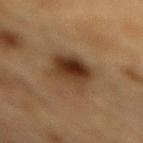Impression:
Imaged during a routine full-body skin examination; the lesion was not biopsied and no histopathology is available.
Context:
The recorded lesion diameter is about 4.5 mm. A region of skin cropped from a whole-body photographic capture, roughly 15 mm wide. From the mid back. The subject is a male aged approximately 85 or older. Captured under cross-polarized illumination. The total-body-photography lesion software estimated a lesion area of about 14 mm², a shape eccentricity near 0.45, and a shape-asymmetry score of about 0.25 (0 = symmetric). And it measured a border-irregularity rating of about 2.5/10, internal color variation of about 8.5 on a 0–10 scale, and a peripheral color-asymmetry measure near 3. And it measured a nevus-likeness score of about 100/100.